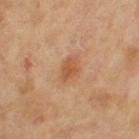  biopsy_status: not biopsied; imaged during a skin examination
  automated_metrics:
    peripheral_color_asymmetry: 0.5
    nevus_likeness_0_100: 65
  patient:
    sex: female
    age_approx: 65
  image:
    source: total-body photography crop
    field_of_view_mm: 15
  lighting: cross-polarized
  site: left upper arm
  lesion_size:
    long_diameter_mm_approx: 3.0